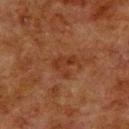The lesion was photographed on a routine skin check and not biopsied; there is no pathology result. The subject is a male roughly 80 years of age. The lesion-visualizer software estimated a border-irregularity rating of about 7/10, a color-variation rating of about 1/10, and a peripheral color-asymmetry measure near 0.5. Approximately 3 mm at its widest. This is a cross-polarized tile. The lesion is on the upper back. A lesion tile, about 15 mm wide, cut from a 3D total-body photograph.